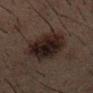notes = imaged on a skin check; not biopsied | imaging modality = total-body-photography crop, ~15 mm field of view | subject = male, approximately 50 years of age | tile lighting = cross-polarized illumination | anatomic site = the mid back.